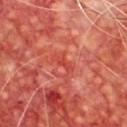This lesion was catalogued during total-body skin photography and was not selected for biopsy.
The lesion is located on the chest.
A 15 mm crop from a total-body photograph taken for skin-cancer surveillance.
A male subject, about 65 years old.
This is a cross-polarized tile.
About 3 mm across.